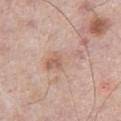Impression:
Recorded during total-body skin imaging; not selected for excision or biopsy.
Image and clinical context:
Automated tile analysis of the lesion measured a border-irregularity index near 5.5/10, a color-variation rating of about 4.5/10, and a peripheral color-asymmetry measure near 1.5. The software also gave a nevus-likeness score of about 0/100. The lesion is located on the right thigh. Approximately 5.5 mm at its widest. Cropped from a total-body skin-imaging series; the visible field is about 15 mm. Imaged with white-light lighting. A male subject, aged around 65.